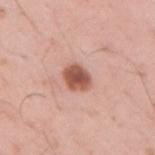Assessment:
Captured during whole-body skin photography for melanoma surveillance; the lesion was not biopsied.
Image and clinical context:
A lesion tile, about 15 mm wide, cut from a 3D total-body photograph. The lesion is located on the right upper arm. A male patient aged 33–37. The tile uses white-light illumination. Approximately 3 mm at its widest.